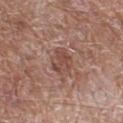Captured during whole-body skin photography for melanoma surveillance; the lesion was not biopsied. Imaged with white-light lighting. The recorded lesion diameter is about 3.5 mm. Located on the leg. A male subject aged 63 to 67. Cropped from a total-body skin-imaging series; the visible field is about 15 mm. The total-body-photography lesion software estimated an average lesion color of about L≈47 a*≈21 b*≈25 (CIELAB) and a normalized border contrast of about 6.